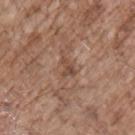Clinical summary:
A male patient aged around 70. From the chest. Captured under white-light illumination. Longest diameter approximately 2.5 mm. A lesion tile, about 15 mm wide, cut from a 3D total-body photograph.